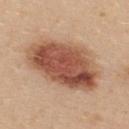biopsy status: catalogued during a skin exam; not biopsied
diameter: ~9.5 mm (longest diameter)
illumination: white-light
acquisition: ~15 mm crop, total-body skin-cancer survey
subject: female, approximately 25 years of age
automated lesion analysis: a footprint of about 38 mm² and two-axis asymmetry of about 0.15; a mean CIELAB color near L≈53 a*≈23 b*≈31, a lesion–skin lightness drop of about 16, and a lesion-to-skin contrast of about 10.5 (normalized; higher = more distinct); a border-irregularity rating of about 2/10 and radial color variation of about 3
location: the upper back